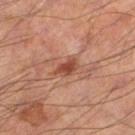Part of a total-body skin-imaging series; this lesion was reviewed on a skin check and was not flagged for biopsy.